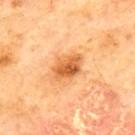The lesion was tiled from a total-body skin photograph and was not biopsied.